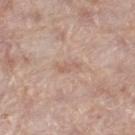biopsy status = no biopsy performed (imaged during a skin exam) | lighting = white-light illumination | acquisition = ~15 mm tile from a whole-body skin photo | location = the right thigh | subject = female, aged 68–72 | lesion diameter = about 3 mm.